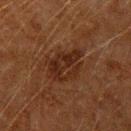Q: Was this lesion biopsied?
A: imaged on a skin check; not biopsied
Q: What kind of image is this?
A: total-body-photography crop, ~15 mm field of view
Q: Illumination type?
A: cross-polarized
Q: What are the patient's age and sex?
A: male, in their 60s
Q: Where on the body is the lesion?
A: the left upper arm
Q: How large is the lesion?
A: ≈4.5 mm
Q: Automated lesion metrics?
A: a mean CIELAB color near L≈20 a*≈16 b*≈23 and a lesion–skin lightness drop of about 6; a border-irregularity rating of about 3.5/10, a color-variation rating of about 4/10, and peripheral color asymmetry of about 1